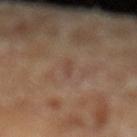This lesion was catalogued during total-body skin photography and was not selected for biopsy.
A male subject, roughly 70 years of age.
Automated tile analysis of the lesion measured an average lesion color of about L≈42 a*≈17 b*≈25 (CIELAB), about 5 CIELAB-L* units darker than the surrounding skin, and a normalized border contrast of about 4.5. The software also gave a lesion-detection confidence of about 75/100.
On the left lower leg.
A 15 mm crop from a total-body photograph taken for skin-cancer surveillance.
Measured at roughly 2.5 mm in maximum diameter.